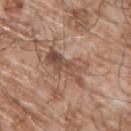{"biopsy_status": "not biopsied; imaged during a skin examination", "site": "upper back", "lesion_size": {"long_diameter_mm_approx": 5.5}, "patient": {"sex": "male", "age_approx": 65}, "image": {"source": "total-body photography crop", "field_of_view_mm": 15}, "automated_metrics": {"area_mm2_approx": 11.0, "eccentricity": 0.9, "shape_asymmetry": 0.35, "cielab_L": 50, "cielab_a": 19, "cielab_b": 27, "vs_skin_darker_L": 10.0}, "lighting": "white-light"}